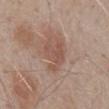Q: What is the imaging modality?
A: total-body-photography crop, ~15 mm field of view
Q: Patient demographics?
A: male, aged 53 to 57
Q: What is the lesion's diameter?
A: about 4 mm
Q: Lesion location?
A: the mid back